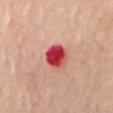The subject is a female aged approximately 60.
A 15 mm close-up extracted from a 3D total-body photography capture.
Automated tile analysis of the lesion measured a lesion color around L≈48 a*≈44 b*≈29 in CIELAB, roughly 19 lightness units darker than nearby skin, and a lesion-to-skin contrast of about 13 (normalized; higher = more distinct). And it measured a peripheral color-asymmetry measure near 2.
Located on the mid back.
Imaged with cross-polarized lighting.
Measured at roughly 3.5 mm in maximum diameter.